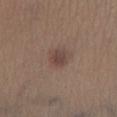{
  "biopsy_status": "not biopsied; imaged during a skin examination",
  "image": {
    "source": "total-body photography crop",
    "field_of_view_mm": 15
  },
  "patient": {
    "sex": "male",
    "age_approx": 35
  },
  "lesion_size": {
    "long_diameter_mm_approx": 2.0
  },
  "site": "right lower leg",
  "automated_metrics": {
    "area_mm2_approx": 3.0,
    "eccentricity": 0.6,
    "shape_asymmetry": 0.25,
    "border_irregularity_0_10": 2.0,
    "color_variation_0_10": 1.5,
    "peripheral_color_asymmetry": 0.5,
    "nevus_likeness_0_100": 65,
    "lesion_detection_confidence_0_100": 100
  },
  "lighting": "white-light"
}